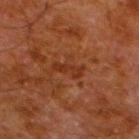Part of a total-body skin-imaging series; this lesion was reviewed on a skin check and was not flagged for biopsy.
On the left lower leg.
This is a cross-polarized tile.
The subject is a male about 80 years old.
A lesion tile, about 15 mm wide, cut from a 3D total-body photograph.
Approximately 3.5 mm at its widest.